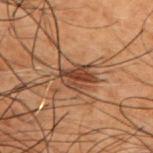biopsy status: total-body-photography surveillance lesion; no biopsy | illumination: cross-polarized | automated metrics: two-axis asymmetry of about 0.4; a lesion color around L≈31 a*≈18 b*≈25 in CIELAB and a normalized border contrast of about 10; a border-irregularity index near 5/10, a within-lesion color-variation index near 3.5/10, and peripheral color asymmetry of about 1; a classifier nevus-likeness of about 25/100 and a lesion-detection confidence of about 75/100 | location: the back | subject: male, roughly 50 years of age | image source: ~15 mm tile from a whole-body skin photo.